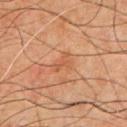– biopsy status: imaged on a skin check; not biopsied
– imaging modality: 15 mm crop, total-body photography
– location: the front of the torso
– patient: male, about 65 years old